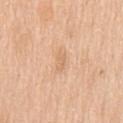notes — imaged on a skin check; not biopsied
acquisition — ~15 mm crop, total-body skin-cancer survey
lesion diameter — about 2.5 mm
TBP lesion metrics — a mean CIELAB color near L≈68 a*≈20 b*≈37, about 7 CIELAB-L* units darker than the surrounding skin, and a lesion-to-skin contrast of about 4.5 (normalized; higher = more distinct); border irregularity of about 3.5 on a 0–10 scale, a within-lesion color-variation index near 0/10, and a peripheral color-asymmetry measure near 0
site — the chest
subject — male, in their 60s